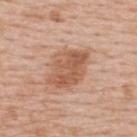Q: What is the anatomic site?
A: the upper back
Q: What is the lesion's diameter?
A: about 5 mm
Q: Automated lesion metrics?
A: an automated nevus-likeness rating near 70 out of 100
Q: How was this image acquired?
A: ~15 mm crop, total-body skin-cancer survey
Q: How was the tile lit?
A: white-light illumination
Q: What are the patient's age and sex?
A: female, roughly 65 years of age